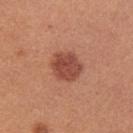lighting — white-light illumination
lesion diameter — ~4 mm (longest diameter)
site — the right upper arm
imaging modality — ~15 mm crop, total-body skin-cancer survey
patient — female, aged approximately 25
TBP lesion metrics — a mean CIELAB color near L≈48 a*≈27 b*≈30, about 12 CIELAB-L* units darker than the surrounding skin, and a normalized lesion–skin contrast near 8.5